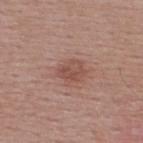Part of a total-body skin-imaging series; this lesion was reviewed on a skin check and was not flagged for biopsy.
A male subject, aged 38 to 42.
Located on the upper back.
Cropped from a total-body skin-imaging series; the visible field is about 15 mm.
This is a white-light tile.
Measured at roughly 3.5 mm in maximum diameter.